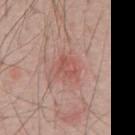biopsy status: no biopsy performed (imaged during a skin exam) | site: the chest | tile lighting: white-light | image source: ~15 mm tile from a whole-body skin photo | patient: male, approximately 70 years of age | lesion diameter: ≈3 mm.